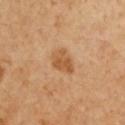biopsy status: no biopsy performed (imaged during a skin exam)
site: the front of the torso
subject: female, aged approximately 55
diameter: ≈3.5 mm
illumination: cross-polarized
automated metrics: an average lesion color of about L≈56 a*≈22 b*≈41 (CIELAB), about 10 CIELAB-L* units darker than the surrounding skin, and a normalized lesion–skin contrast near 7.5; border irregularity of about 3.5 on a 0–10 scale, a color-variation rating of about 3/10, and radial color variation of about 1
image: 15 mm crop, total-body photography